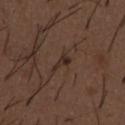Captured during whole-body skin photography for melanoma surveillance; the lesion was not biopsied. Imaged with white-light lighting. A roughly 15 mm field-of-view crop from a total-body skin photograph. About 3 mm across. From the abdomen. A male subject, aged 48–52.